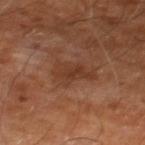No biopsy was performed on this lesion — it was imaged during a full skin examination and was not determined to be concerning.
A roughly 15 mm field-of-view crop from a total-body skin photograph.
The lesion is located on the right thigh.
The subject is in their mid- to late 60s.
Measured at roughly 5 mm in maximum diameter.
The tile uses cross-polarized illumination.